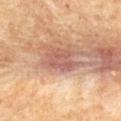Findings:
* workup · no biopsy performed (imaged during a skin exam)
* acquisition · total-body-photography crop, ~15 mm field of view
* body site · the chest
* automated lesion analysis · a lesion area of about 7.5 mm² and a symmetry-axis asymmetry near 0.3
* subject · male, aged 63–67
* lighting · cross-polarized illumination